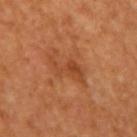| field | value |
|---|---|
| workup | imaged on a skin check; not biopsied |
| location | the arm |
| patient | female, in their mid- to late 50s |
| image | ~15 mm tile from a whole-body skin photo |